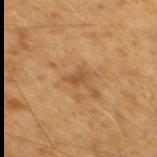Captured during whole-body skin photography for melanoma surveillance; the lesion was not biopsied.
The lesion's longest dimension is about 2.5 mm.
This image is a 15 mm lesion crop taken from a total-body photograph.
Captured under cross-polarized illumination.
Located on the upper back.
A male subject, about 60 years old.